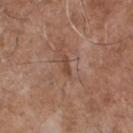The lesion was photographed on a routine skin check and not biopsied; there is no pathology result. The tile uses white-light illumination. About 2.5 mm across. This image is a 15 mm lesion crop taken from a total-body photograph. On the chest. A male subject aged around 70.